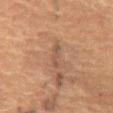<tbp_lesion>
  <biopsy_status>not biopsied; imaged during a skin examination</biopsy_status>
  <automated_metrics>
    <cielab_L>43</cielab_L>
    <cielab_a>16</cielab_a>
    <cielab_b>25</cielab_b>
    <vs_skin_contrast_norm>5.5</vs_skin_contrast_norm>
    <border_irregularity_0_10>6.0</border_irregularity_0_10>
    <color_variation_0_10>0.0</color_variation_0_10>
    <peripheral_color_asymmetry>0.0</peripheral_color_asymmetry>
    <lesion_detection_confidence_0_100>65</lesion_detection_confidence_0_100>
  </automated_metrics>
  <patient>
    <sex>female</sex>
    <age_approx>70</age_approx>
  </patient>
  <lesion_size>
    <long_diameter_mm_approx>3.0</long_diameter_mm_approx>
  </lesion_size>
  <site>abdomen</site>
  <image>
    <source>total-body photography crop</source>
    <field_of_view_mm>15</field_of_view_mm>
  </image>
</tbp_lesion>